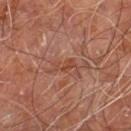Impression:
This lesion was catalogued during total-body skin photography and was not selected for biopsy.
Clinical summary:
Located on the leg. A close-up tile cropped from a whole-body skin photograph, about 15 mm across. About 3 mm across. Captured under cross-polarized illumination. The subject is a male about 60 years old.